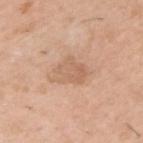Impression:
Captured during whole-body skin photography for melanoma surveillance; the lesion was not biopsied.
Background:
An algorithmic analysis of the crop reported a lesion area of about 8.5 mm² and an outline eccentricity of about 0.55 (0 = round, 1 = elongated). And it measured a lesion color around L≈62 a*≈19 b*≈32 in CIELAB, roughly 8 lightness units darker than nearby skin, and a normalized border contrast of about 5. Captured under white-light illumination. Cropped from a whole-body photographic skin survey; the tile spans about 15 mm. A male patient, aged approximately 50. On the left upper arm.